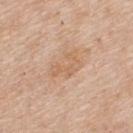Notes:
– workup: no biopsy performed (imaged during a skin exam)
– acquisition: ~15 mm crop, total-body skin-cancer survey
– tile lighting: white-light
– anatomic site: the upper back
– automated metrics: an average lesion color of about L≈63 a*≈19 b*≈34 (CIELAB), about 7 CIELAB-L* units darker than the surrounding skin, and a lesion-to-skin contrast of about 5 (normalized; higher = more distinct); a color-variation rating of about 0/10 and a peripheral color-asymmetry measure near 0
– patient: male, approximately 55 years of age